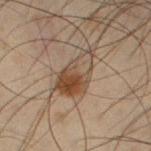Captured during whole-body skin photography for melanoma surveillance; the lesion was not biopsied. A roughly 15 mm field-of-view crop from a total-body skin photograph. Approximately 5.5 mm at its widest. Imaged with cross-polarized lighting. A male subject aged 48 to 52. The lesion is located on the right forearm.